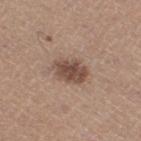Clinical impression:
Imaged during a routine full-body skin examination; the lesion was not biopsied and no histopathology is available.
Image and clinical context:
Automated image analysis of the tile measured a lesion–skin lightness drop of about 12. It also reported a nevus-likeness score of about 60/100 and a lesion-detection confidence of about 100/100. Measured at roughly 4 mm in maximum diameter. Cropped from a whole-body photographic skin survey; the tile spans about 15 mm. The lesion is located on the right thigh. A female patient approximately 45 years of age. Captured under white-light illumination.